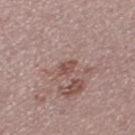Clinical impression:
No biopsy was performed on this lesion — it was imaged during a full skin examination and was not determined to be concerning.
Clinical summary:
A female patient, roughly 25 years of age. A lesion tile, about 15 mm wide, cut from a 3D total-body photograph. On the left thigh.